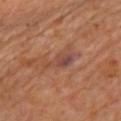Assessment:
This lesion was catalogued during total-body skin photography and was not selected for biopsy.
Background:
The lesion is located on the front of the torso. The recorded lesion diameter is about 4.5 mm. A 15 mm crop from a total-body photograph taken for skin-cancer surveillance. The patient is a female aged approximately 60.